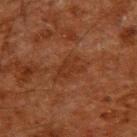imaging modality=total-body-photography crop, ~15 mm field of view; body site=the upper back; tile lighting=cross-polarized illumination; automated metrics=a within-lesion color-variation index near 1/10; lesion size=~3.5 mm (longest diameter); subject=male, aged around 60.